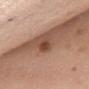About 3 mm across. A close-up tile cropped from a whole-body skin photograph, about 15 mm across. A female patient, aged 53 to 57. The lesion is located on the chest. This is a white-light tile.